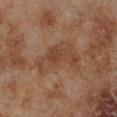tile lighting = cross-polarized
location = the left lower leg
subject = male, aged 68 to 72
image-analysis metrics = an area of roughly 17 mm², an eccentricity of roughly 0.8, and two-axis asymmetry of about 0.4; a lesion color around L≈42 a*≈19 b*≈29 in CIELAB and a lesion–skin lightness drop of about 6; a border-irregularity rating of about 4.5/10 and a color-variation rating of about 4/10
size = ~6 mm (longest diameter)
image = ~15 mm tile from a whole-body skin photo